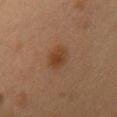Longest diameter approximately 3 mm.
On the chest.
The tile uses cross-polarized illumination.
A female subject, approximately 40 years of age.
A lesion tile, about 15 mm wide, cut from a 3D total-body photograph.